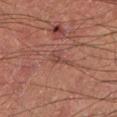Clinical impression: Captured during whole-body skin photography for melanoma surveillance; the lesion was not biopsied. Background: The subject is a male in their mid-50s. From the left lower leg. This is a cross-polarized tile. This image is a 15 mm lesion crop taken from a total-body photograph.